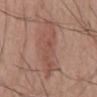* biopsy status · total-body-photography surveillance lesion; no biopsy
* tile lighting · white-light
* acquisition · total-body-photography crop, ~15 mm field of view
* subject · male, aged 53–57
* TBP lesion metrics · a footprint of about 17 mm², an eccentricity of roughly 0.95, and two-axis asymmetry of about 0.3; a mean CIELAB color near L≈51 a*≈22 b*≈26; border irregularity of about 6 on a 0–10 scale, a color-variation rating of about 3/10, and a peripheral color-asymmetry measure near 1; a nevus-likeness score of about 5/100 and a lesion-detection confidence of about 95/100
* location · the mid back